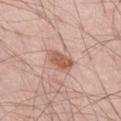Q: Is there a histopathology result?
A: catalogued during a skin exam; not biopsied
Q: What is the anatomic site?
A: the right thigh
Q: How was the tile lit?
A: white-light illumination
Q: Who is the patient?
A: male, aged approximately 55
Q: What kind of image is this?
A: ~15 mm crop, total-body skin-cancer survey